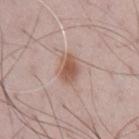Case summary:
– biopsy status: imaged on a skin check; not biopsied
– imaging modality: ~15 mm crop, total-body skin-cancer survey
– patient: male, approximately 70 years of age
– body site: the chest
– lesion diameter: about 4 mm